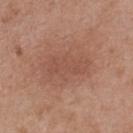Recorded during total-body skin imaging; not selected for excision or biopsy.
Measured at roughly 5.5 mm in maximum diameter.
A female patient, aged around 40.
A roughly 15 mm field-of-view crop from a total-body skin photograph.
Located on the upper back.
Captured under white-light illumination.